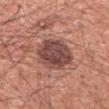Case summary:
• biopsy status · imaged on a skin check; not biopsied
• subject · male, aged approximately 60
• body site · the arm
• acquisition · ~15 mm crop, total-body skin-cancer survey